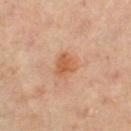workup: total-body-photography surveillance lesion; no biopsy
image: ~15 mm crop, total-body skin-cancer survey
automated lesion analysis: a nevus-likeness score of about 85/100
subject: female, aged 58–62
size: about 3 mm
illumination: cross-polarized
anatomic site: the right lower leg